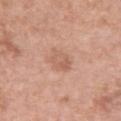Recorded during total-body skin imaging; not selected for excision or biopsy. Located on the upper back. A region of skin cropped from a whole-body photographic capture, roughly 15 mm wide. A female subject, about 60 years old. Captured under white-light illumination.